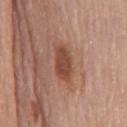follow-up = imaged on a skin check; not biopsied | patient = male, about 80 years old | body site = the front of the torso | imaging modality = total-body-photography crop, ~15 mm field of view | size = about 4.5 mm | illumination = white-light.